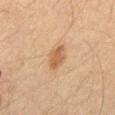follow-up = no biopsy performed (imaged during a skin exam) | patient = male, aged 63–67 | image source = 15 mm crop, total-body photography | lesion diameter = ~3 mm (longest diameter) | automated lesion analysis = a lesion area of about 5.5 mm², a shape eccentricity near 0.75, and two-axis asymmetry of about 0.2; a mean CIELAB color near L≈48 a*≈17 b*≈32, about 9 CIELAB-L* units darker than the surrounding skin, and a normalized lesion–skin contrast near 7.5; lesion-presence confidence of about 100/100 | location = the mid back | tile lighting = cross-polarized illumination.